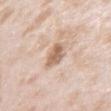Q: Was this lesion biopsied?
A: total-body-photography surveillance lesion; no biopsy
Q: How was this image acquired?
A: ~15 mm crop, total-body skin-cancer survey
Q: What lighting was used for the tile?
A: white-light illumination
Q: Who is the patient?
A: female, aged 23–27
Q: Where on the body is the lesion?
A: the left upper arm